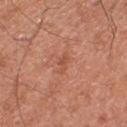{"biopsy_status": "not biopsied; imaged during a skin examination", "automated_metrics": {"vs_skin_darker_L": 7.0, "vs_skin_contrast_norm": 5.0, "border_irregularity_0_10": 3.5, "color_variation_0_10": 0.0}, "lesion_size": {"long_diameter_mm_approx": 2.5}, "image": {"source": "total-body photography crop", "field_of_view_mm": 15}, "site": "front of the torso", "lighting": "white-light", "patient": {"sex": "male", "age_approx": 65}}